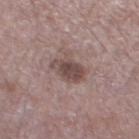Part of a total-body skin-imaging series; this lesion was reviewed on a skin check and was not flagged for biopsy.
Imaged with white-light lighting.
The subject is a male approximately 70 years of age.
Automated tile analysis of the lesion measured a lesion color around L≈46 a*≈16 b*≈19 in CIELAB, roughly 11 lightness units darker than nearby skin, and a lesion-to-skin contrast of about 8.5 (normalized; higher = more distinct). The analysis additionally found internal color variation of about 3 on a 0–10 scale and radial color variation of about 1.
The lesion is on the left thigh.
Longest diameter approximately 3.5 mm.
A region of skin cropped from a whole-body photographic capture, roughly 15 mm wide.